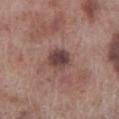{"biopsy_status": "not biopsied; imaged during a skin examination", "lighting": "white-light", "site": "right lower leg", "image": {"source": "total-body photography crop", "field_of_view_mm": 15}, "lesion_size": {"long_diameter_mm_approx": 3.5}, "patient": {"sex": "male", "age_approx": 70}}